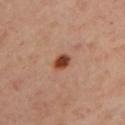Q: Was a biopsy performed?
A: catalogued during a skin exam; not biopsied
Q: Automated lesion metrics?
A: a border-irregularity rating of about 1/10 and a peripheral color-asymmetry measure near 1
Q: What is the lesion's diameter?
A: about 2 mm
Q: What kind of image is this?
A: ~15 mm tile from a whole-body skin photo
Q: Illumination type?
A: cross-polarized illumination
Q: Patient demographics?
A: female, aged 38 to 42
Q: Lesion location?
A: the left upper arm